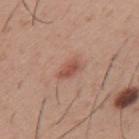Part of a total-body skin-imaging series; this lesion was reviewed on a skin check and was not flagged for biopsy. Cropped from a whole-body photographic skin survey; the tile spans about 15 mm. From the upper back. A male subject, aged 28 to 32. This is a white-light tile. The lesion's longest dimension is about 2.5 mm.